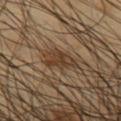biopsy_status: not biopsied; imaged during a skin examination
image:
  source: total-body photography crop
  field_of_view_mm: 15
automated_metrics:
  color_variation_0_10: 2.5
  peripheral_color_asymmetry: 1.0
lesion_size:
  long_diameter_mm_approx: 3.5
patient:
  sex: male
  age_approx: 40
site: front of the torso
lighting: cross-polarized